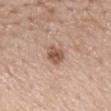• notes · imaged on a skin check; not biopsied
• body site · the mid back
• tile lighting · white-light illumination
• acquisition · ~15 mm tile from a whole-body skin photo
• patient · male, aged 63–67
• lesion diameter · ≈2.5 mm